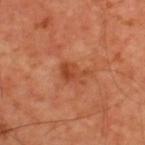No biopsy was performed on this lesion — it was imaged during a full skin examination and was not determined to be concerning.
This is a cross-polarized tile.
A 15 mm crop from a total-body photograph taken for skin-cancer surveillance.
About 3.5 mm across.
A male subject roughly 60 years of age.
An algorithmic analysis of the crop reported a footprint of about 5 mm² and a shape eccentricity near 0.8. And it measured a nevus-likeness score of about 5/100.
From the upper back.